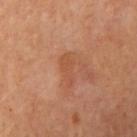Imaged during a routine full-body skin examination; the lesion was not biopsied and no histopathology is available. The lesion is located on the right upper arm. A female subject, aged around 70. A region of skin cropped from a whole-body photographic capture, roughly 15 mm wide. Measured at roughly 3.5 mm in maximum diameter. Captured under cross-polarized illumination.